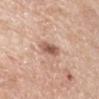Captured during whole-body skin photography for melanoma surveillance; the lesion was not biopsied.
On the left upper arm.
Measured at roughly 2.5 mm in maximum diameter.
The patient is a male roughly 70 years of age.
A 15 mm close-up tile from a total-body photography series done for melanoma screening.
This is a white-light tile.